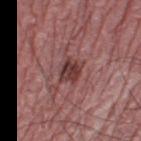Part of a total-body skin-imaging series; this lesion was reviewed on a skin check and was not flagged for biopsy. A region of skin cropped from a whole-body photographic capture, roughly 15 mm wide. Automated tile analysis of the lesion measured a color-variation rating of about 4/10 and a peripheral color-asymmetry measure near 1.5. It also reported a nevus-likeness score of about 30/100 and lesion-presence confidence of about 100/100. A male subject about 75 years old. Approximately 3 mm at its widest. On the left lower leg.